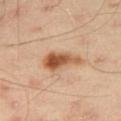<lesion>
  <biopsy_status>not biopsied; imaged during a skin examination</biopsy_status>
</lesion>